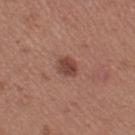Context: A 15 mm close-up extracted from a 3D total-body photography capture. Automated image analysis of the tile measured a border-irregularity index near 2/10 and internal color variation of about 3 on a 0–10 scale. It also reported an automated nevus-likeness rating near 85 out of 100 and lesion-presence confidence of about 100/100. The lesion is on the left thigh. The patient is a female roughly 30 years of age. Imaged with white-light lighting. The lesion's longest dimension is about 3 mm.